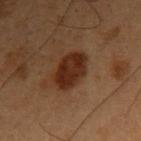Background: This image is a 15 mm lesion crop taken from a total-body photograph. A male subject in their mid-50s. From the right upper arm. Imaged with cross-polarized lighting. The recorded lesion diameter is about 4 mm.